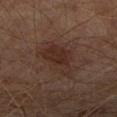  biopsy_status: not biopsied; imaged during a skin examination
  patient:
    sex: male
    age_approx: 65
  lesion_size:
    long_diameter_mm_approx: 6.0
  automated_metrics:
    cielab_L: 28
    cielab_a: 17
    cielab_b: 22
    vs_skin_darker_L: 7.0
    vs_skin_contrast_norm: 7.0
    border_irregularity_0_10: 3.5
    color_variation_0_10: 3.0
    peripheral_color_asymmetry: 1.0
  image:
    source: total-body photography crop
    field_of_view_mm: 15
  site: right lower leg